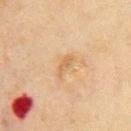workup = no biopsy performed (imaged during a skin exam)
TBP lesion metrics = an area of roughly 4 mm², a shape eccentricity near 0.9, and a symmetry-axis asymmetry near 0.3; an automated nevus-likeness rating near 0 out of 100 and lesion-presence confidence of about 100/100
diameter = ≈3 mm
imaging modality = 15 mm crop, total-body photography
tile lighting = cross-polarized
body site = the chest
subject = male, about 70 years old